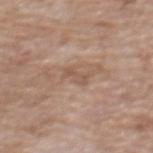follow-up: total-body-photography surveillance lesion; no biopsy
tile lighting: white-light
TBP lesion metrics: an area of roughly 3 mm² and an outline eccentricity of about 0.9 (0 = round, 1 = elongated); a nevus-likeness score of about 0/100 and a detector confidence of about 100 out of 100 that the crop contains a lesion
body site: the chest
subject: male, aged 58–62
imaging modality: total-body-photography crop, ~15 mm field of view
size: about 3 mm